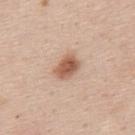workup = catalogued during a skin exam; not biopsied
anatomic site = the upper back
subject = male, about 45 years old
diameter = about 3 mm
illumination = white-light
image source = ~15 mm crop, total-body skin-cancer survey